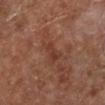The lesion's longest dimension is about 2.5 mm. A roughly 15 mm field-of-view crop from a total-body skin photograph. The lesion is located on the left lower leg. A patient aged approximately 65.